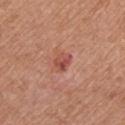No biopsy was performed on this lesion — it was imaged during a full skin examination and was not determined to be concerning. Cropped from a whole-body photographic skin survey; the tile spans about 15 mm. On the arm. A female subject, in their mid- to late 60s. Captured under white-light illumination.